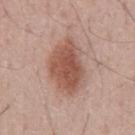workup: catalogued during a skin exam; not biopsied
lighting: white-light
lesion diameter: ~6.5 mm (longest diameter)
patient: male, in their mid-50s
imaging modality: total-body-photography crop, ~15 mm field of view